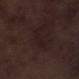This lesion was catalogued during total-body skin photography and was not selected for biopsy. About 4 mm across. On the left lower leg. The patient is a male in their 70s. Automated tile analysis of the lesion measured a lesion–skin lightness drop of about 3 and a normalized border contrast of about 5. The analysis additionally found a nevus-likeness score of about 0/100 and a lesion-detection confidence of about 50/100. A lesion tile, about 15 mm wide, cut from a 3D total-body photograph.